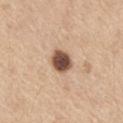Captured during whole-body skin photography for melanoma surveillance; the lesion was not biopsied. A 15 mm close-up tile from a total-body photography series done for melanoma screening. A male subject approximately 70 years of age. On the abdomen.